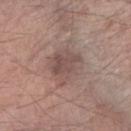  biopsy_status: not biopsied; imaged during a skin examination
  lesion_size:
    long_diameter_mm_approx: 4.0
  site: arm
  patient:
    sex: male
    age_approx: 80
  automated_metrics:
    area_mm2_approx: 12.0
    eccentricity: 0.4
    border_irregularity_0_10: 2.5
  image:
    source: total-body photography crop
    field_of_view_mm: 15
  lighting: white-light